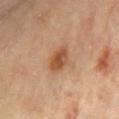biopsy status: imaged on a skin check; not biopsied
image source: 15 mm crop, total-body photography
site: the chest
size: ~3.5 mm (longest diameter)
automated metrics: an area of roughly 6 mm², an eccentricity of roughly 0.8, and a symmetry-axis asymmetry near 0.15; a mean CIELAB color near L≈51 a*≈23 b*≈35, roughly 11 lightness units darker than nearby skin, and a normalized lesion–skin contrast near 8.5; a border-irregularity index near 1.5/10, internal color variation of about 3.5 on a 0–10 scale, and radial color variation of about 1
subject: female, aged approximately 60